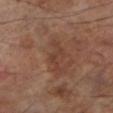{"biopsy_status": "not biopsied; imaged during a skin examination", "lighting": "cross-polarized", "lesion_size": {"long_diameter_mm_approx": 4.5}, "site": "left lower leg", "image": {"source": "total-body photography crop", "field_of_view_mm": 15}, "patient": {"sex": "male", "age_approx": 70}, "automated_metrics": {"area_mm2_approx": 5.0, "eccentricity": 0.95, "shape_asymmetry": 0.65, "vs_skin_darker_L": 6.0, "vs_skin_contrast_norm": 5.5}}